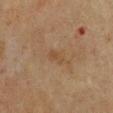- size: ~2.5 mm (longest diameter)
- image: ~15 mm tile from a whole-body skin photo
- patient: female, approximately 55 years of age
- location: the chest